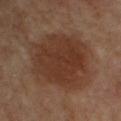Q: Was this lesion biopsied?
A: no biopsy performed (imaged during a skin exam)
Q: How large is the lesion?
A: ≈7.5 mm
Q: What kind of image is this?
A: total-body-photography crop, ~15 mm field of view
Q: Lesion location?
A: the right upper arm
Q: Who is the patient?
A: male, aged around 70
Q: What did automated image analysis measure?
A: a lesion area of about 42 mm², an outline eccentricity of about 0.4 (0 = round, 1 = elongated), and a shape-asymmetry score of about 0.15 (0 = symmetric); a border-irregularity rating of about 2/10, a color-variation rating of about 3.5/10, and peripheral color asymmetry of about 1; an automated nevus-likeness rating near 80 out of 100 and a detector confidence of about 100 out of 100 that the crop contains a lesion
Q: How was the tile lit?
A: cross-polarized